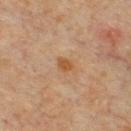No biopsy was performed on this lesion — it was imaged during a full skin examination and was not determined to be concerning. A 15 mm crop from a total-body photograph taken for skin-cancer surveillance. The lesion's longest dimension is about 2.5 mm. A male patient aged around 65. Imaged with cross-polarized lighting. The lesion is located on the front of the torso.